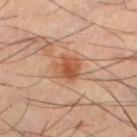The lesion was tiled from a total-body skin photograph and was not biopsied. A 15 mm close-up extracted from a 3D total-body photography capture. A male patient aged around 40. From the left lower leg. About 3.5 mm across. Imaged with cross-polarized lighting.